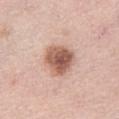Located on the left thigh.
About 4 mm across.
Automated image analysis of the tile measured an average lesion color of about L≈58 a*≈21 b*≈28 (CIELAB), about 16 CIELAB-L* units darker than the surrounding skin, and a lesion-to-skin contrast of about 10 (normalized; higher = more distinct). It also reported a border-irregularity index near 2/10, a color-variation rating of about 5.5/10, and peripheral color asymmetry of about 1.5.
A female subject, about 65 years old.
This image is a 15 mm lesion crop taken from a total-body photograph.